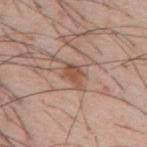Cropped from a total-body skin-imaging series; the visible field is about 15 mm. From the mid back. The subject is a male in their mid-50s.